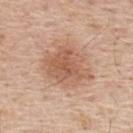Part of a total-body skin-imaging series; this lesion was reviewed on a skin check and was not flagged for biopsy. Cropped from a total-body skin-imaging series; the visible field is about 15 mm. The subject is a male aged 53–57. Located on the back. The tile uses white-light illumination. Automated tile analysis of the lesion measured a border-irregularity rating of about 3/10, a within-lesion color-variation index near 4/10, and a peripheral color-asymmetry measure near 1. About 6 mm across.